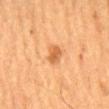{
  "biopsy_status": "not biopsied; imaged during a skin examination",
  "automated_metrics": {
    "eccentricity": 0.7,
    "shape_asymmetry": 0.25
  },
  "image": {
    "source": "total-body photography crop",
    "field_of_view_mm": 15
  },
  "lighting": "cross-polarized",
  "patient": {
    "sex": "male",
    "age_approx": 65
  },
  "site": "mid back"
}